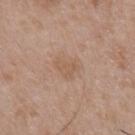- notes: catalogued during a skin exam; not biopsied
- image source: ~15 mm tile from a whole-body skin photo
- lesion diameter: ~3 mm (longest diameter)
- subject: male, about 65 years old
- illumination: white-light
- image-analysis metrics: a lesion–skin lightness drop of about 5 and a normalized border contrast of about 4.5; a border-irregularity rating of about 4/10 and peripheral color asymmetry of about 0.5; a nevus-likeness score of about 0/100 and a lesion-detection confidence of about 100/100
- body site: the chest